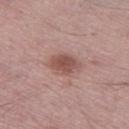automated lesion analysis: a footprint of about 8.5 mm² and an outline eccentricity of about 0.75 (0 = round, 1 = elongated); an average lesion color of about L≈52 a*≈21 b*≈23 (CIELAB), a lesion–skin lightness drop of about 11, and a normalized lesion–skin contrast near 7.5; border irregularity of about 2 on a 0–10 scale, a within-lesion color-variation index near 3/10, and a peripheral color-asymmetry measure near 1; a nevus-likeness score of about 70/100 and lesion-presence confidence of about 100/100 | anatomic site: the left thigh | subject: male, in their 50s | tile lighting: white-light | lesion size: about 4 mm | image source: ~15 mm tile from a whole-body skin photo.